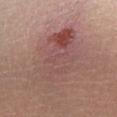biopsy status: catalogued during a skin exam; not biopsied | image source: ~15 mm tile from a whole-body skin photo | location: the left lower leg | patient: male, in their mid-40s | diameter: ~8 mm (longest diameter).